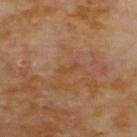biopsy status: no biopsy performed (imaged during a skin exam) | image source: ~15 mm tile from a whole-body skin photo | lesion diameter: about 2.5 mm | subject: male, about 70 years old | automated lesion analysis: a footprint of about 2 mm², an outline eccentricity of about 0.95 (0 = round, 1 = elongated), and a shape-asymmetry score of about 0.5 (0 = symmetric); a border-irregularity index near 5.5/10, internal color variation of about 0 on a 0–10 scale, and radial color variation of about 0; a nevus-likeness score of about 0/100 and a lesion-detection confidence of about 100/100 | location: the upper back | illumination: cross-polarized illumination.